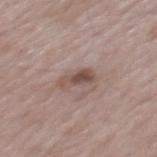Clinical impression: Imaged during a routine full-body skin examination; the lesion was not biopsied and no histopathology is available. Clinical summary: A male subject roughly 65 years of age. A 15 mm close-up tile from a total-body photography series done for melanoma screening. The lesion is on the mid back.